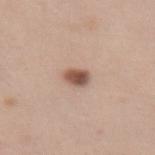<tbp_lesion>
  <biopsy_status>not biopsied; imaged during a skin examination</biopsy_status>
  <automated_metrics>
    <border_irregularity_0_10>1.5</border_irregularity_0_10>
    <color_variation_0_10>4.5</color_variation_0_10>
  </automated_metrics>
  <lesion_size>
    <long_diameter_mm_approx>2.5</long_diameter_mm_approx>
  </lesion_size>
  <image>
    <source>total-body photography crop</source>
    <field_of_view_mm>15</field_of_view_mm>
  </image>
  <site>leg</site>
  <lighting>white-light</lighting>
  <patient>
    <sex>female</sex>
    <age_approx>40</age_approx>
  </patient>
</tbp_lesion>